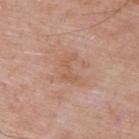Acquisition and patient details:
From the upper back. The total-body-photography lesion software estimated border irregularity of about 6 on a 0–10 scale and a within-lesion color-variation index near 0.5/10. The tile uses white-light illumination. The subject is a male in their mid-60s. Approximately 3.5 mm at its widest. A 15 mm crop from a total-body photograph taken for skin-cancer surveillance.